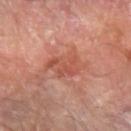| key | value |
|---|---|
| notes | imaged on a skin check; not biopsied |
| site | the left forearm |
| subject | male, aged approximately 70 |
| tile lighting | cross-polarized |
| lesion diameter | ~5 mm (longest diameter) |
| acquisition | ~15 mm crop, total-body skin-cancer survey |